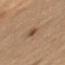<case>
  <biopsy_status>not biopsied; imaged during a skin examination</biopsy_status>
  <automated_metrics>
    <area_mm2_approx>3.0</area_mm2_approx>
    <vs_skin_darker_L>10.0</vs_skin_darker_L>
    <vs_skin_contrast_norm>7.5</vs_skin_contrast_norm>
    <border_irregularity_0_10>2.5</border_irregularity_0_10>
    <color_variation_0_10>1.0</color_variation_0_10>
  </automated_metrics>
  <image>
    <source>total-body photography crop</source>
    <field_of_view_mm>15</field_of_view_mm>
  </image>
  <lesion_size>
    <long_diameter_mm_approx>3.0</long_diameter_mm_approx>
  </lesion_size>
  <patient>
    <sex>male</sex>
    <age_approx>70</age_approx>
  </patient>
  <site>arm</site>
  <lighting>white-light</lighting>
</case>